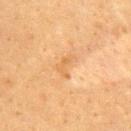Assessment:
This lesion was catalogued during total-body skin photography and was not selected for biopsy.
Image and clinical context:
Longest diameter approximately 3 mm. A female subject about 40 years old. Cropped from a whole-body photographic skin survey; the tile spans about 15 mm. This is a cross-polarized tile. The total-body-photography lesion software estimated a mean CIELAB color near L≈58 a*≈20 b*≈40, a lesion–skin lightness drop of about 6, and a normalized border contrast of about 5. The analysis additionally found border irregularity of about 4.5 on a 0–10 scale, internal color variation of about 1.5 on a 0–10 scale, and peripheral color asymmetry of about 0.5. And it measured a classifier nevus-likeness of about 0/100 and a lesion-detection confidence of about 100/100. From the back.